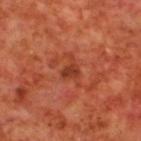Imaged during a routine full-body skin examination; the lesion was not biopsied and no histopathology is available. On the back. The recorded lesion diameter is about 3 mm. A roughly 15 mm field-of-view crop from a total-body skin photograph. This is a cross-polarized tile. The subject is a male aged 68 to 72.